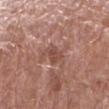This lesion was catalogued during total-body skin photography and was not selected for biopsy.
A region of skin cropped from a whole-body photographic capture, roughly 15 mm wide.
A male subject, aged approximately 80.
From the right forearm.